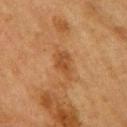Case summary:
* follow-up · total-body-photography surveillance lesion; no biopsy
* body site · the right upper arm
* automated metrics · a classifier nevus-likeness of about 0/100 and a detector confidence of about 100 out of 100 that the crop contains a lesion
* patient · female, roughly 55 years of age
* image source · 15 mm crop, total-body photography
* size · about 3.5 mm